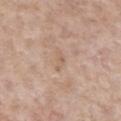No biopsy was performed on this lesion — it was imaged during a full skin examination and was not determined to be concerning. The lesion is on the front of the torso. A female patient aged around 85 or older. A roughly 15 mm field-of-view crop from a total-body skin photograph. Imaged with white-light lighting. About 2.5 mm across.